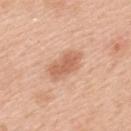Findings:
- follow-up — catalogued during a skin exam; not biopsied
- lesion size — ≈4.5 mm
- lighting — white-light
- acquisition — 15 mm crop, total-body photography
- subject — male, aged around 50
- anatomic site — the mid back